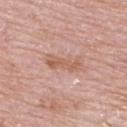Impression: Part of a total-body skin-imaging series; this lesion was reviewed on a skin check and was not flagged for biopsy. Clinical summary: A region of skin cropped from a whole-body photographic capture, roughly 15 mm wide. Approximately 4.5 mm at its widest. The lesion is on the upper back. The patient is a female approximately 65 years of age. This is a white-light tile.